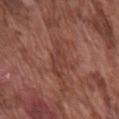image source=total-body-photography crop, ~15 mm field of view; location=the right upper arm; patient=male, aged 73 to 77.